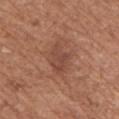Notes:
– biopsy status · no biopsy performed (imaged during a skin exam)
– acquisition · ~15 mm tile from a whole-body skin photo
– anatomic site · the upper back
– subject · female, about 75 years old
– automated metrics · an area of roughly 8.5 mm², a shape eccentricity near 0.75, and a symmetry-axis asymmetry near 0.2; an average lesion color of about L≈46 a*≈22 b*≈28 (CIELAB) and a normalized border contrast of about 6
– lighting · white-light